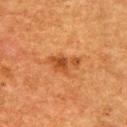biopsy status — catalogued during a skin exam; not biopsied | subject — female, approximately 50 years of age | location — the right upper arm | acquisition — 15 mm crop, total-body photography.